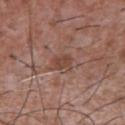Clinical impression:
Recorded during total-body skin imaging; not selected for excision or biopsy.
Acquisition and patient details:
About 2.5 mm across. Cropped from a whole-body photographic skin survey; the tile spans about 15 mm. The lesion is located on the chest. A male patient, aged approximately 45. Imaged with white-light lighting.